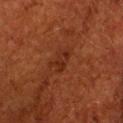– subject · female, in their 50s
– illumination · cross-polarized illumination
– automated metrics · a lesion color around L≈23 a*≈22 b*≈27 in CIELAB, about 5 CIELAB-L* units darker than the surrounding skin, and a normalized border contrast of about 6
– site · the head or neck
– lesion size · ~3 mm (longest diameter)
– imaging modality · total-body-photography crop, ~15 mm field of view